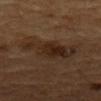Case summary:
* workup · no biopsy performed (imaged during a skin exam)
* imaging modality · 15 mm crop, total-body photography
* subject · male, in their mid- to late 80s or older
* lighting · cross-polarized
* anatomic site · the upper back